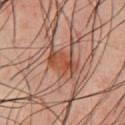| feature | finding |
|---|---|
| workup | catalogued during a skin exam; not biopsied |
| automated lesion analysis | an automated nevus-likeness rating near 45 out of 100 |
| imaging modality | ~15 mm crop, total-body skin-cancer survey |
| subject | male, roughly 50 years of age |
| illumination | cross-polarized illumination |
| anatomic site | the chest |